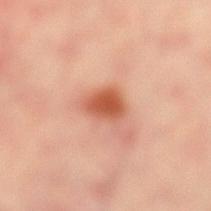biopsy status — no biopsy performed (imaged during a skin exam)
imaging modality — ~15 mm crop, total-body skin-cancer survey
site — the right leg
diameter — ≈3.5 mm
automated metrics — a lesion area of about 6.5 mm², an outline eccentricity of about 0.7 (0 = round, 1 = elongated), and a shape-asymmetry score of about 0.2 (0 = symmetric); an average lesion color of about L≈58 a*≈30 b*≈36 (CIELAB), a lesion–skin lightness drop of about 14, and a lesion-to-skin contrast of about 9 (normalized; higher = more distinct); a border-irregularity rating of about 2/10, a within-lesion color-variation index near 4/10, and radial color variation of about 1
tile lighting — cross-polarized
subject — female, about 40 years old